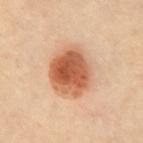follow-up: no biopsy performed (imaged during a skin exam); body site: the chest; subject: female, about 55 years old; image-analysis metrics: a nevus-likeness score of about 100/100 and a detector confidence of about 100 out of 100 that the crop contains a lesion; lesion size: ~5.5 mm (longest diameter); illumination: cross-polarized illumination; imaging modality: ~15 mm tile from a whole-body skin photo.